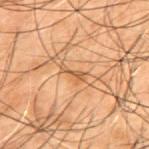follow-up — total-body-photography surveillance lesion; no biopsy | image-analysis metrics — a footprint of about 3 mm² and a symmetry-axis asymmetry near 0.45; peripheral color asymmetry of about 0; a classifier nevus-likeness of about 0/100 and a detector confidence of about 55 out of 100 that the crop contains a lesion | imaging modality — ~15 mm crop, total-body skin-cancer survey | subject — male, approximately 50 years of age | site — the upper back | tile lighting — cross-polarized.